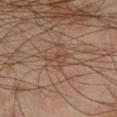| key | value |
|---|---|
| biopsy status | total-body-photography surveillance lesion; no biopsy |
| patient | male, in their mid- to late 40s |
| automated metrics | two-axis asymmetry of about 0.55; a mean CIELAB color near L≈36 a*≈15 b*≈23; a border-irregularity rating of about 6/10, a color-variation rating of about 2/10, and peripheral color asymmetry of about 1; a classifier nevus-likeness of about 0/100 and a detector confidence of about 90 out of 100 that the crop contains a lesion |
| acquisition | ~15 mm tile from a whole-body skin photo |
| anatomic site | the right lower leg |